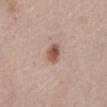<record>
  <biopsy_status>not biopsied; imaged during a skin examination</biopsy_status>
  <site>abdomen</site>
  <image>
    <source>total-body photography crop</source>
    <field_of_view_mm>15</field_of_view_mm>
  </image>
  <lighting>white-light</lighting>
  <lesion_size>
    <long_diameter_mm_approx>3.0</long_diameter_mm_approx>
  </lesion_size>
  <patient>
    <sex>male</sex>
    <age_approx>65</age_approx>
  </patient>
</record>